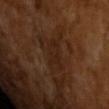Q: Is there a histopathology result?
A: imaged on a skin check; not biopsied
Q: What is the lesion's diameter?
A: about 3.5 mm
Q: Patient demographics?
A: male, aged 63–67
Q: What is the imaging modality?
A: 15 mm crop, total-body photography
Q: How was the tile lit?
A: cross-polarized
Q: What did automated image analysis measure?
A: an eccentricity of roughly 0.75 and a shape-asymmetry score of about 0.4 (0 = symmetric); a lesion color around L≈21 a*≈17 b*≈24 in CIELAB and a normalized lesion–skin contrast near 4.5; a nevus-likeness score of about 0/100 and lesion-presence confidence of about 80/100